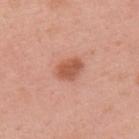Imaged during a routine full-body skin examination; the lesion was not biopsied and no histopathology is available. A female patient, aged around 50. Measured at roughly 3.5 mm in maximum diameter. A 15 mm crop from a total-body photograph taken for skin-cancer surveillance. On the back. Imaged with white-light lighting.